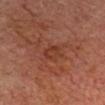{
  "biopsy_status": "not biopsied; imaged during a skin examination",
  "site": "head or neck",
  "lesion_size": {
    "long_diameter_mm_approx": 3.0
  },
  "lighting": "cross-polarized",
  "patient": {
    "sex": "male",
    "age_approx": 75
  },
  "image": {
    "source": "total-body photography crop",
    "field_of_view_mm": 15
  }
}